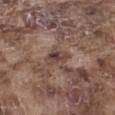Imaged during a routine full-body skin examination; the lesion was not biopsied and no histopathology is available.
A male subject, in their mid-70s.
The lesion's longest dimension is about 2.5 mm.
On the left thigh.
A 15 mm close-up tile from a total-body photography series done for melanoma screening.
Captured under white-light illumination.